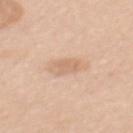Assessment:
Part of a total-body skin-imaging series; this lesion was reviewed on a skin check and was not flagged for biopsy.
Acquisition and patient details:
A 15 mm crop from a total-body photograph taken for skin-cancer surveillance. The lesion is on the mid back. This is a white-light tile. A female patient, aged around 60. Approximately 3 mm at its widest.